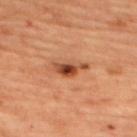Imaged during a routine full-body skin examination; the lesion was not biopsied and no histopathology is available. The lesion's longest dimension is about 4 mm. A female subject aged 43 to 47. Located on the upper back. Cropped from a whole-body photographic skin survey; the tile spans about 15 mm.